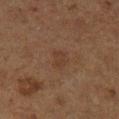acquisition: ~15 mm crop, total-body skin-cancer survey | body site: the left lower leg | tile lighting: cross-polarized illumination | patient: male, aged approximately 75 | automated metrics: a lesion area of about 4.5 mm² and a shape-asymmetry score of about 0.25 (0 = symmetric); border irregularity of about 2.5 on a 0–10 scale, internal color variation of about 1.5 on a 0–10 scale, and a peripheral color-asymmetry measure near 0.5; a nevus-likeness score of about 0/100 and lesion-presence confidence of about 100/100.